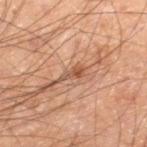anatomic site = the right lower leg | imaging modality = ~15 mm tile from a whole-body skin photo | patient = male, in their mid-60s | illumination = cross-polarized | automated metrics = a footprint of about 2.5 mm² and two-axis asymmetry of about 0.5; about 10 CIELAB-L* units darker than the surrounding skin and a normalized lesion–skin contrast near 7.5; a border-irregularity rating of about 5.5/10, internal color variation of about 0.5 on a 0–10 scale, and radial color variation of about 0.